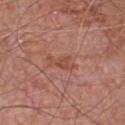The lesion was photographed on a routine skin check and not biopsied; there is no pathology result. The lesion is on the chest. A male patient in their mid- to late 60s. Measured at roughly 3.5 mm in maximum diameter. A 15 mm close-up extracted from a 3D total-body photography capture. The tile uses white-light illumination.